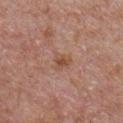The lesion was tiled from a total-body skin photograph and was not biopsied.
The lesion is on the chest.
The lesion's longest dimension is about 2.5 mm.
The patient is a male approximately 65 years of age.
This image is a 15 mm lesion crop taken from a total-body photograph.